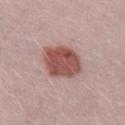follow-up — catalogued during a skin exam; not biopsied | subject — male, in their 30s | tile lighting — white-light illumination | automated metrics — a mean CIELAB color near L≈52 a*≈24 b*≈23 and a normalized border contrast of about 10 | image — 15 mm crop, total-body photography.